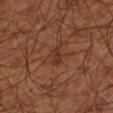The lesion was photographed on a routine skin check and not biopsied; there is no pathology result. The lesion is located on the left lower leg. A patient roughly 65 years of age. A 15 mm crop from a total-body photograph taken for skin-cancer surveillance.